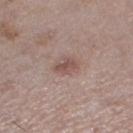Findings:
- biopsy status · catalogued during a skin exam; not biopsied
- lesion size · about 2.5 mm
- imaging modality · 15 mm crop, total-body photography
- location · the left thigh
- subject · female, aged approximately 60